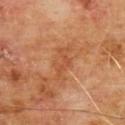Assessment:
Captured during whole-body skin photography for melanoma surveillance; the lesion was not biopsied.
Clinical summary:
Automated tile analysis of the lesion measured an area of roughly 5.5 mm², an eccentricity of roughly 0.9, and a symmetry-axis asymmetry near 0.5. The analysis additionally found a mean CIELAB color near L≈49 a*≈26 b*≈37, roughly 7 lightness units darker than nearby skin, and a lesion-to-skin contrast of about 5 (normalized; higher = more distinct). The software also gave a lesion-detection confidence of about 100/100. The recorded lesion diameter is about 4.5 mm. A 15 mm close-up tile from a total-body photography series done for melanoma screening. A male subject, aged 58–62. On the chest.